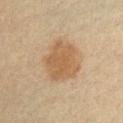Captured during whole-body skin photography for melanoma surveillance; the lesion was not biopsied.
A male patient, approximately 40 years of age.
Captured under cross-polarized illumination.
From the right upper arm.
The recorded lesion diameter is about 5 mm.
Automated tile analysis of the lesion measured a footprint of about 17 mm², an eccentricity of roughly 0.5, and a symmetry-axis asymmetry near 0.2. It also reported a mean CIELAB color near L≈52 a*≈16 b*≈33, about 9 CIELAB-L* units darker than the surrounding skin, and a lesion-to-skin contrast of about 7 (normalized; higher = more distinct).
Cropped from a whole-body photographic skin survey; the tile spans about 15 mm.